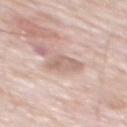<lesion>
  <biopsy_status>not biopsied; imaged during a skin examination</biopsy_status>
  <lighting>white-light</lighting>
  <lesion_size>
    <long_diameter_mm_approx>3.5</long_diameter_mm_approx>
  </lesion_size>
  <site>back</site>
  <patient>
    <sex>male</sex>
    <age_approx>80</age_approx>
  </patient>
  <automated_metrics>
    <eccentricity>0.65</eccentricity>
    <shape_asymmetry>0.35</shape_asymmetry>
    <cielab_L>64</cielab_L>
    <cielab_a>17</cielab_a>
    <cielab_b>25</cielab_b>
    <vs_skin_darker_L>10.0</vs_skin_darker_L>
    <vs_skin_contrast_norm>6.0</vs_skin_contrast_norm>
  </automated_metrics>
  <image>
    <source>total-body photography crop</source>
    <field_of_view_mm>15</field_of_view_mm>
  </image>
</lesion>